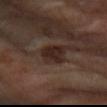workup: no biopsy performed (imaged during a skin exam) | subject: female, aged approximately 60 | automated metrics: a lesion area of about 5.5 mm², an outline eccentricity of about 0.55 (0 = round, 1 = elongated), and a shape-asymmetry score of about 0.4 (0 = symmetric) | image: 15 mm crop, total-body photography | location: the right forearm | illumination: cross-polarized | lesion size: ≈3 mm.